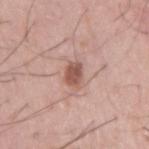No biopsy was performed on this lesion — it was imaged during a full skin examination and was not determined to be concerning. Longest diameter approximately 3 mm. A male subject, aged 53 to 57. The lesion-visualizer software estimated a nevus-likeness score of about 90/100 and a detector confidence of about 100 out of 100 that the crop contains a lesion. A close-up tile cropped from a whole-body skin photograph, about 15 mm across.